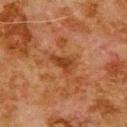The lesion-visualizer software estimated a symmetry-axis asymmetry near 0.4. The software also gave border irregularity of about 4.5 on a 0–10 scale and radial color variation of about 0.5. It also reported lesion-presence confidence of about 100/100. The subject is a male about 80 years old. The lesion is located on the upper back. Cropped from a total-body skin-imaging series; the visible field is about 15 mm. About 3 mm across. Imaged with cross-polarized lighting.